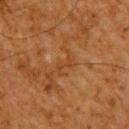| field | value |
|---|---|
| biopsy status | catalogued during a skin exam; not biopsied |
| body site | the upper back |
| patient | male, aged 58 to 62 |
| acquisition | ~15 mm tile from a whole-body skin photo |
| size | ≈2.5 mm |
| illumination | cross-polarized illumination |